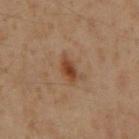biopsy status: imaged on a skin check; not biopsied
automated lesion analysis: a mean CIELAB color near L≈39 a*≈20 b*≈30 and a normalized border contrast of about 8.5; a border-irregularity index near 3/10 and internal color variation of about 3 on a 0–10 scale; a nevus-likeness score of about 85/100 and a detector confidence of about 100 out of 100 that the crop contains a lesion
lighting: cross-polarized illumination
image: ~15 mm crop, total-body skin-cancer survey
anatomic site: the mid back
lesion size: ~3.5 mm (longest diameter)
patient: male, roughly 60 years of age